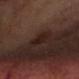Assessment: The lesion was tiled from a total-body skin photograph and was not biopsied. Background: A 15 mm close-up extracted from a 3D total-body photography capture. A male subject about 65 years old. The recorded lesion diameter is about 3 mm. An algorithmic analysis of the crop reported an area of roughly 4 mm², a shape eccentricity near 0.85, and a symmetry-axis asymmetry near 0.25. And it measured a mean CIELAB color near L≈19 a*≈15 b*≈20, about 6 CIELAB-L* units darker than the surrounding skin, and a normalized lesion–skin contrast near 8. The software also gave an automated nevus-likeness rating near 0 out of 100 and a lesion-detection confidence of about 95/100. The tile uses cross-polarized illumination.